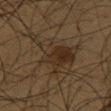Q: Was this lesion biopsied?
A: total-body-photography surveillance lesion; no biopsy
Q: Lesion location?
A: the chest
Q: How was this image acquired?
A: ~15 mm tile from a whole-body skin photo
Q: Who is the patient?
A: male, aged around 60
Q: Automated lesion metrics?
A: a lesion color around L≈22 a*≈9 b*≈20 in CIELAB and a normalized border contrast of about 6.5